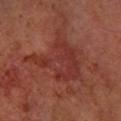biopsy_status: not biopsied; imaged during a skin examination
patient:
  sex: male
  age_approx: 60
image:
  source: total-body photography crop
  field_of_view_mm: 15
site: right forearm